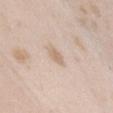biopsy status: imaged on a skin check; not biopsied | tile lighting: white-light | subject: female, in their mid-20s | automated lesion analysis: a normalized lesion–skin contrast near 6 | image: ~15 mm tile from a whole-body skin photo | body site: the chest.